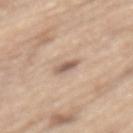Impression: This lesion was catalogued during total-body skin photography and was not selected for biopsy. Clinical summary: The subject is a male aged 68–72. Longest diameter approximately 2.5 mm. A 15 mm close-up tile from a total-body photography series done for melanoma screening. Located on the mid back.